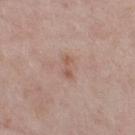notes: catalogued during a skin exam; not biopsied | tile lighting: white-light illumination | acquisition: ~15 mm tile from a whole-body skin photo | body site: the right thigh | patient: female, aged around 65.